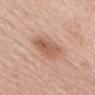Notes:
* notes: imaged on a skin check; not biopsied
* diameter: about 4.5 mm
* subject: female, approximately 60 years of age
* lighting: white-light illumination
* location: the mid back
* image source: 15 mm crop, total-body photography